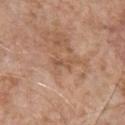<lesion>
  <biopsy_status>not biopsied; imaged during a skin examination</biopsy_status>
  <image>
    <source>total-body photography crop</source>
    <field_of_view_mm>15</field_of_view_mm>
  </image>
  <lighting>white-light</lighting>
  <site>chest</site>
  <lesion_size>
    <long_diameter_mm_approx>2.5</long_diameter_mm_approx>
  </lesion_size>
  <patient>
    <sex>male</sex>
    <age_approx>55</age_approx>
  </patient>
</lesion>